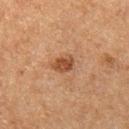follow-up — imaged on a skin check; not biopsied | site — the leg | image — total-body-photography crop, ~15 mm field of view | image-analysis metrics — an average lesion color of about L≈38 a*≈20 b*≈29 (CIELAB) and about 10 CIELAB-L* units darker than the surrounding skin; a nevus-likeness score of about 85/100 and a detector confidence of about 100 out of 100 that the crop contains a lesion | subject — male, in their mid- to late 70s | diameter — ≈3 mm.